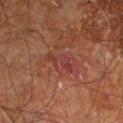Assessment:
Part of a total-body skin-imaging series; this lesion was reviewed on a skin check and was not flagged for biopsy.
Image and clinical context:
Imaged with cross-polarized lighting. Longest diameter approximately 4 mm. An algorithmic analysis of the crop reported an area of roughly 5.5 mm², an outline eccentricity of about 0.9 (0 = round, 1 = elongated), and a symmetry-axis asymmetry near 0.4. It also reported a lesion color around L≈38 a*≈27 b*≈25 in CIELAB, a lesion–skin lightness drop of about 6, and a lesion-to-skin contrast of about 6 (normalized; higher = more distinct). The software also gave a border-irregularity rating of about 5.5/10, a within-lesion color-variation index near 2/10, and radial color variation of about 0.5. On the leg. Cropped from a total-body skin-imaging series; the visible field is about 15 mm. A male patient, in their 60s.